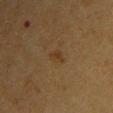Case summary:
* follow-up: no biopsy performed (imaged during a skin exam)
* image source: total-body-photography crop, ~15 mm field of view
* body site: the right upper arm
* patient: female, aged approximately 55
* size: ~2.5 mm (longest diameter)